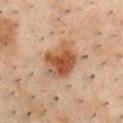Q: Is there a histopathology result?
A: catalogued during a skin exam; not biopsied
Q: What is the imaging modality?
A: 15 mm crop, total-body photography
Q: Where on the body is the lesion?
A: the front of the torso
Q: Illumination type?
A: cross-polarized
Q: Patient demographics?
A: male, aged around 50